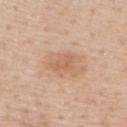Located on the upper back.
The tile uses white-light illumination.
Longest diameter approximately 2.5 mm.
A 15 mm close-up tile from a total-body photography series done for melanoma screening.
The patient is a male aged approximately 60.